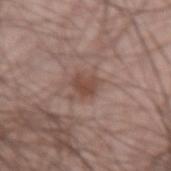biopsy status = catalogued during a skin exam; not biopsied
subject = male, roughly 65 years of age
image source = ~15 mm tile from a whole-body skin photo
anatomic site = the left forearm